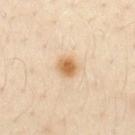The lesion was photographed on a routine skin check and not biopsied; there is no pathology result.
From the chest.
Automated image analysis of the tile measured a footprint of about 4.5 mm² and a symmetry-axis asymmetry near 0.15. The software also gave a border-irregularity index near 1/10 and a within-lesion color-variation index near 3/10. And it measured an automated nevus-likeness rating near 100 out of 100 and lesion-presence confidence of about 100/100.
Captured under cross-polarized illumination.
A male patient, aged 28 to 32.
Cropped from a whole-body photographic skin survey; the tile spans about 15 mm.